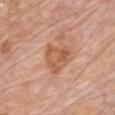Notes:
– notes · catalogued during a skin exam; not biopsied
– size · about 4 mm
– lighting · white-light
– imaging modality · total-body-photography crop, ~15 mm field of view
– anatomic site · the chest
– subject · male, aged around 80
– image-analysis metrics · about 9 CIELAB-L* units darker than the surrounding skin and a lesion-to-skin contrast of about 6.5 (normalized; higher = more distinct)